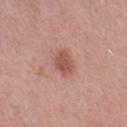Impression:
Imaged during a routine full-body skin examination; the lesion was not biopsied and no histopathology is available.
Background:
The subject is a female aged 38 to 42. An algorithmic analysis of the crop reported an area of roughly 6 mm², an eccentricity of roughly 0.7, and two-axis asymmetry of about 0.25. The software also gave an average lesion color of about L≈53 a*≈24 b*≈27 (CIELAB), a lesion–skin lightness drop of about 10, and a lesion-to-skin contrast of about 7.5 (normalized; higher = more distinct). It also reported a color-variation rating of about 2/10 and radial color variation of about 0.5. The analysis additionally found a classifier nevus-likeness of about 70/100 and a detector confidence of about 100 out of 100 that the crop contains a lesion. A 15 mm close-up tile from a total-body photography series done for melanoma screening. Longest diameter approximately 3 mm. Captured under white-light illumination. The lesion is located on the right thigh.